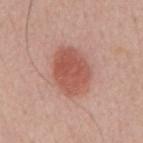{"biopsy_status": "not biopsied; imaged during a skin examination", "automated_metrics": {"cielab_L": 55, "cielab_a": 26, "cielab_b": 28, "vs_skin_darker_L": 11.0, "border_irregularity_0_10": 1.5, "color_variation_0_10": 3.5}, "lesion_size": {"long_diameter_mm_approx": 5.5}, "site": "mid back", "lighting": "white-light", "image": {"source": "total-body photography crop", "field_of_view_mm": 15}, "patient": {"sex": "male", "age_approx": 55}}